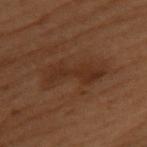The recorded lesion diameter is about 7 mm. A 15 mm close-up extracted from a 3D total-body photography capture. On the back. The patient is a female aged around 50. The tile uses cross-polarized illumination.